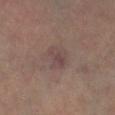The lesion was photographed on a routine skin check and not biopsied; there is no pathology result. A 15 mm close-up tile from a total-body photography series done for melanoma screening. The recorded lesion diameter is about 3 mm. An algorithmic analysis of the crop reported a within-lesion color-variation index near 3/10 and a peripheral color-asymmetry measure near 1. And it measured an automated nevus-likeness rating near 0 out of 100 and a lesion-detection confidence of about 100/100. A male patient in their mid-60s. The lesion is located on the right lower leg.